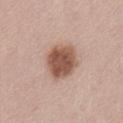The patient is a male roughly 55 years of age. A region of skin cropped from a whole-body photographic capture, roughly 15 mm wide. The lesion is on the lower back.